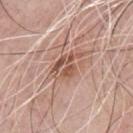* notes — total-body-photography surveillance lesion; no biopsy
* subject — male, aged 63 to 67
* image — 15 mm crop, total-body photography
* body site — the chest